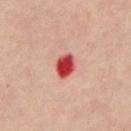The lesion is on the abdomen.
The patient is a male aged approximately 65.
A lesion tile, about 15 mm wide, cut from a 3D total-body photograph.